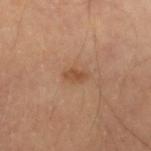– workup — total-body-photography surveillance lesion; no biopsy
– site — the left forearm
– subject — male, approximately 70 years of age
– illumination — cross-polarized illumination
– acquisition — ~15 mm crop, total-body skin-cancer survey